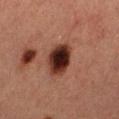Notes:
- biopsy status — catalogued during a skin exam; not biopsied
- anatomic site — the right thigh
- image source — total-body-photography crop, ~15 mm field of view
- patient — female, aged approximately 40
- diameter — ~4 mm (longest diameter)